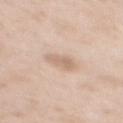{"biopsy_status": "not biopsied; imaged during a skin examination", "patient": {"sex": "female", "age_approx": 50}, "image": {"source": "total-body photography crop", "field_of_view_mm": 15}, "lighting": "white-light", "site": "upper back"}